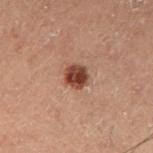biopsy status: no biopsy performed (imaged during a skin exam)
image: total-body-photography crop, ~15 mm field of view
lesion size: ≈2.5 mm
anatomic site: the left thigh
lighting: cross-polarized
subject: male, in their 60s